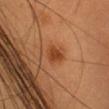Case summary:
- workup · no biopsy performed (imaged during a skin exam)
- image-analysis metrics · an average lesion color of about L≈44 a*≈27 b*≈38 (CIELAB), about 10 CIELAB-L* units darker than the surrounding skin, and a normalized border contrast of about 7.5
- tile lighting · cross-polarized illumination
- lesion diameter · ≈3 mm
- body site · the head or neck
- subject · female, approximately 35 years of age
- acquisition · ~15 mm crop, total-body skin-cancer survey